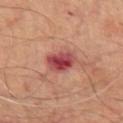Findings:
- biopsy status · no biopsy performed (imaged during a skin exam)
- tile lighting · cross-polarized illumination
- image-analysis metrics · an area of roughly 7.5 mm² and two-axis asymmetry of about 0.2; a lesion color around L≈45 a*≈33 b*≈23 in CIELAB, about 14 CIELAB-L* units darker than the surrounding skin, and a normalized border contrast of about 11; an automated nevus-likeness rating near 0 out of 100 and a detector confidence of about 100 out of 100 that the crop contains a lesion
- image · ~15 mm crop, total-body skin-cancer survey
- diameter · about 3.5 mm
- anatomic site · the chest
- subject · male, in their mid- to late 60s